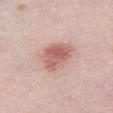Q: Is there a histopathology result?
A: catalogued during a skin exam; not biopsied
Q: What is the imaging modality?
A: total-body-photography crop, ~15 mm field of view
Q: What is the anatomic site?
A: the chest
Q: Who is the patient?
A: female, approximately 60 years of age
Q: What lighting was used for the tile?
A: white-light illumination
Q: Automated lesion metrics?
A: a lesion area of about 12 mm² and a symmetry-axis asymmetry near 0.2; border irregularity of about 2 on a 0–10 scale, a color-variation rating of about 4.5/10, and peripheral color asymmetry of about 1.5; a nevus-likeness score of about 85/100 and a detector confidence of about 100 out of 100 that the crop contains a lesion